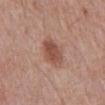notes = no biopsy performed (imaged during a skin exam); subject = male, in their mid- to late 50s; location = the mid back; illumination = white-light; image = 15 mm crop, total-body photography; size = about 4 mm; image-analysis metrics = a lesion area of about 9 mm² and an eccentricity of roughly 0.65.